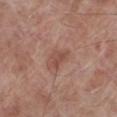The lesion was tiled from a total-body skin photograph and was not biopsied. From the left lower leg. A 15 mm close-up tile from a total-body photography series done for melanoma screening. A male patient, roughly 70 years of age. The lesion's longest dimension is about 2.5 mm.